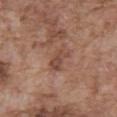Image and clinical context:
This image is a 15 mm lesion crop taken from a total-body photograph. The tile uses white-light illumination. A male subject aged approximately 75. The lesion is on the abdomen.